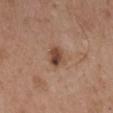  biopsy_status: not biopsied; imaged during a skin examination
  lesion_size:
    long_diameter_mm_approx: 2.5
  site: mid back
  patient:
    sex: male
    age_approx: 55
  image:
    source: total-body photography crop
    field_of_view_mm: 15
  lighting: white-light